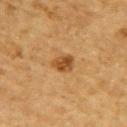No biopsy was performed on this lesion — it was imaged during a full skin examination and was not determined to be concerning. On the back. Captured under cross-polarized illumination. A male patient about 85 years old or older. Approximately 2.5 mm at its widest. A roughly 15 mm field-of-view crop from a total-body skin photograph. The lesion-visualizer software estimated two-axis asymmetry of about 0.2. The software also gave a lesion-to-skin contrast of about 9 (normalized; higher = more distinct). The software also gave a border-irregularity rating of about 2/10, a within-lesion color-variation index near 4/10, and peripheral color asymmetry of about 1.5. And it measured a classifier nevus-likeness of about 90/100 and a lesion-detection confidence of about 100/100.